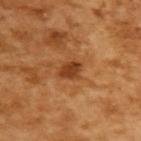patient — male, approximately 65 years of age | image — ~15 mm crop, total-body skin-cancer survey.